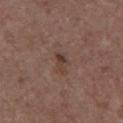No biopsy was performed on this lesion — it was imaged during a full skin examination and was not determined to be concerning. The subject is a male about 60 years old. Imaged with white-light lighting. A region of skin cropped from a whole-body photographic capture, roughly 15 mm wide. The lesion is located on the chest. Measured at roughly 2.5 mm in maximum diameter.